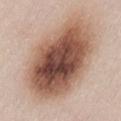The subject is a female aged around 75.
Automated tile analysis of the lesion measured an average lesion color of about L≈53 a*≈20 b*≈28 (CIELAB), roughly 19 lightness units darker than nearby skin, and a lesion-to-skin contrast of about 12.5 (normalized; higher = more distinct). It also reported internal color variation of about 9.5 on a 0–10 scale and a peripheral color-asymmetry measure near 3. The analysis additionally found a nevus-likeness score of about 90/100 and lesion-presence confidence of about 100/100.
From the lower back.
A 15 mm close-up tile from a total-body photography series done for melanoma screening.
Imaged with white-light lighting.